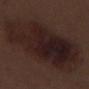Q: Lesion size?
A: ≈14 mm
Q: Lesion location?
A: the right lower leg
Q: What is the imaging modality?
A: 15 mm crop, total-body photography
Q: Automated lesion metrics?
A: a mean CIELAB color near L≈20 a*≈15 b*≈16, about 8 CIELAB-L* units darker than the surrounding skin, and a lesion-to-skin contrast of about 10 (normalized; higher = more distinct); an automated nevus-likeness rating near 40 out of 100 and a lesion-detection confidence of about 100/100
Q: What are the patient's age and sex?
A: male, aged around 70
Q: What lighting was used for the tile?
A: white-light illumination